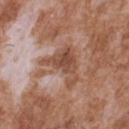Findings:
- automated metrics · a footprint of about 13 mm², a shape eccentricity near 0.7, and two-axis asymmetry of about 0.65; a mean CIELAB color near L≈50 a*≈21 b*≈29, about 10 CIELAB-L* units darker than the surrounding skin, and a normalized lesion–skin contrast near 7.5; a border-irregularity rating of about 7/10 and a color-variation rating of about 4.5/10; a nevus-likeness score of about 0/100
- anatomic site · the back
- subject · male, aged around 45
- acquisition · ~15 mm tile from a whole-body skin photo
- size · ~5 mm (longest diameter)